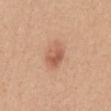{"biopsy_status": "not biopsied; imaged during a skin examination", "patient": {"sex": "female", "age_approx": 55}, "automated_metrics": {"area_mm2_approx": 6.5, "eccentricity": 0.7, "shape_asymmetry": 0.25, "border_irregularity_0_10": 2.5, "color_variation_0_10": 5.0, "peripheral_color_asymmetry": 2.0}, "site": "chest", "image": {"source": "total-body photography crop", "field_of_view_mm": 15}}